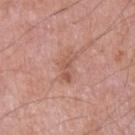Findings:
– patient: male, in their 50s
– tile lighting: white-light illumination
– anatomic site: the right upper arm
– acquisition: total-body-photography crop, ~15 mm field of view